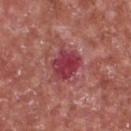notes = imaged on a skin check; not biopsied | location = the front of the torso | lighting = white-light | patient = male, aged approximately 65 | size = ~4.5 mm (longest diameter) | acquisition = total-body-photography crop, ~15 mm field of view.